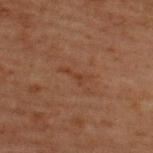Clinical impression:
No biopsy was performed on this lesion — it was imaged during a full skin examination and was not determined to be concerning.
Context:
A 15 mm crop from a total-body photograph taken for skin-cancer surveillance. A male patient, aged 58–62. Measured at roughly 2.5 mm in maximum diameter. The lesion is on the back. This is a cross-polarized tile.